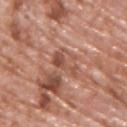Cropped from a total-body skin-imaging series; the visible field is about 15 mm.
Imaged with white-light lighting.
The lesion is on the upper back.
A male subject, aged approximately 70.